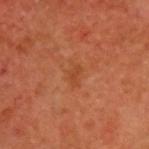notes = no biopsy performed (imaged during a skin exam) | acquisition = ~15 mm tile from a whole-body skin photo | lighting = cross-polarized illumination | body site = the upper back | patient = male, approximately 60 years of age.